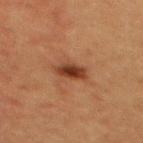This lesion was catalogued during total-body skin photography and was not selected for biopsy.
A 15 mm crop from a total-body photograph taken for skin-cancer surveillance.
A female patient, aged 38 to 42.
An algorithmic analysis of the crop reported an area of roughly 5.5 mm², a shape eccentricity near 0.75, and two-axis asymmetry of about 0.2. The analysis additionally found a classifier nevus-likeness of about 95/100.
Imaged with cross-polarized lighting.
On the mid back.
Approximately 3.5 mm at its widest.